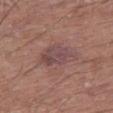Case summary:
– biopsy status — no biopsy performed (imaged during a skin exam)
– location — the right thigh
– image-analysis metrics — a lesion area of about 9.5 mm², a shape eccentricity near 0.6, and a symmetry-axis asymmetry near 0.3; a classifier nevus-likeness of about 0/100
– acquisition — ~15 mm crop, total-body skin-cancer survey
– tile lighting — white-light illumination
– lesion diameter — ~4 mm (longest diameter)
– subject — male, aged 68–72